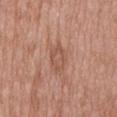workup: imaged on a skin check; not biopsied | subject: male, aged around 50 | illumination: white-light | body site: the mid back | automated metrics: a shape-asymmetry score of about 0.3 (0 = symmetric) | image: ~15 mm tile from a whole-body skin photo.